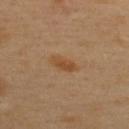notes: total-body-photography surveillance lesion; no biopsy
subject: male, aged 38 to 42
imaging modality: ~15 mm tile from a whole-body skin photo
size: ~3.5 mm (longest diameter)
anatomic site: the upper back